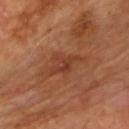| field | value |
|---|---|
| follow-up | no biopsy performed (imaged during a skin exam) |
| lesion diameter | ~3.5 mm (longest diameter) |
| site | the back |
| image source | total-body-photography crop, ~15 mm field of view |
| lighting | cross-polarized |
| TBP lesion metrics | an average lesion color of about L≈38 a*≈24 b*≈31 (CIELAB), roughly 7 lightness units darker than nearby skin, and a lesion-to-skin contrast of about 6 (normalized; higher = more distinct); a border-irregularity index near 5.5/10, internal color variation of about 3 on a 0–10 scale, and a peripheral color-asymmetry measure near 1; a nevus-likeness score of about 0/100 and a detector confidence of about 100 out of 100 that the crop contains a lesion |
| patient | male, roughly 60 years of age |